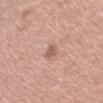Part of a total-body skin-imaging series; this lesion was reviewed on a skin check and was not flagged for biopsy.
Longest diameter approximately 2.5 mm.
From the right upper arm.
The tile uses white-light illumination.
A 15 mm close-up tile from a total-body photography series done for melanoma screening.
A female patient in their mid-30s.